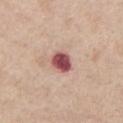Q: Illumination type?
A: white-light illumination
Q: What did automated image analysis measure?
A: a nevus-likeness score of about 0/100 and a detector confidence of about 100 out of 100 that the crop contains a lesion
Q: How was this image acquired?
A: 15 mm crop, total-body photography
Q: What is the lesion's diameter?
A: ~3 mm (longest diameter)
Q: Lesion location?
A: the chest
Q: What are the patient's age and sex?
A: male, about 60 years old